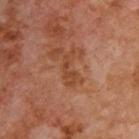A male patient approximately 70 years of age.
A 15 mm close-up tile from a total-body photography series done for melanoma screening.
Captured under cross-polarized illumination.
Located on the upper back.
The lesion-visualizer software estimated a lesion color around L≈43 a*≈24 b*≈33 in CIELAB and a lesion-to-skin contrast of about 6.5 (normalized; higher = more distinct). The analysis additionally found a classifier nevus-likeness of about 0/100 and a lesion-detection confidence of about 100/100.
About 4 mm across.